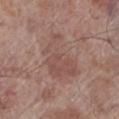Notes:
– follow-up: no biopsy performed (imaged during a skin exam)
– location: the left lower leg
– patient: male, aged approximately 70
– acquisition: 15 mm crop, total-body photography